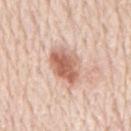Part of a total-body skin-imaging series; this lesion was reviewed on a skin check and was not flagged for biopsy. Measured at roughly 4.5 mm in maximum diameter. Located on the mid back. A region of skin cropped from a whole-body photographic capture, roughly 15 mm wide. Captured under white-light illumination. The total-body-photography lesion software estimated a mean CIELAB color near L≈62 a*≈23 b*≈30 and a normalized border contrast of about 9. The subject is a male aged around 80.